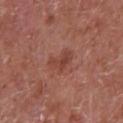notes: no biopsy performed (imaged during a skin exam) | subject: male, aged 63–67 | image: ~15 mm tile from a whole-body skin photo | tile lighting: white-light illumination | site: the chest | size: ~2.5 mm (longest diameter) | image-analysis metrics: a footprint of about 3.5 mm² and an outline eccentricity of about 0.8 (0 = round, 1 = elongated); border irregularity of about 5.5 on a 0–10 scale, internal color variation of about 0.5 on a 0–10 scale, and radial color variation of about 0; an automated nevus-likeness rating near 10 out of 100 and a lesion-detection confidence of about 100/100.